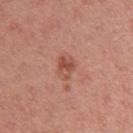biopsy_status: not biopsied; imaged during a skin examination
patient:
  sex: male
  age_approx: 40
image:
  source: total-body photography crop
  field_of_view_mm: 15
site: left upper arm
lighting: white-light